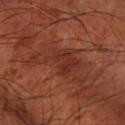Q: Was this lesion biopsied?
A: imaged on a skin check; not biopsied
Q: What is the imaging modality?
A: 15 mm crop, total-body photography
Q: Lesion location?
A: the left forearm
Q: Who is the patient?
A: in their mid- to late 60s
Q: How was the tile lit?
A: cross-polarized